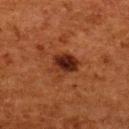{
  "biopsy_status": "not biopsied; imaged during a skin examination",
  "patient": {
    "sex": "female",
    "age_approx": 50
  },
  "image": {
    "source": "total-body photography crop",
    "field_of_view_mm": 15
  },
  "lesion_size": {
    "long_diameter_mm_approx": 3.5
  },
  "lighting": "cross-polarized",
  "site": "upper back"
}